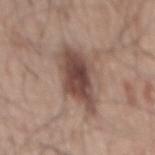Assessment: Captured during whole-body skin photography for melanoma surveillance; the lesion was not biopsied. Context: A male subject aged 48 to 52. The lesion's longest dimension is about 6.5 mm. A 15 mm crop from a total-body photograph taken for skin-cancer surveillance. On the back.